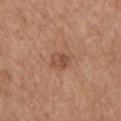notes: imaged on a skin check; not biopsied | image source: ~15 mm crop, total-body skin-cancer survey | image-analysis metrics: an eccentricity of roughly 0.65 and two-axis asymmetry of about 0.2; a border-irregularity index near 2/10, internal color variation of about 4.5 on a 0–10 scale, and peripheral color asymmetry of about 2 | patient: male, in their mid- to late 60s | site: the left upper arm | lesion size: ≈2.5 mm.